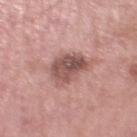Image and clinical context:
From the left lower leg. The recorded lesion diameter is about 5 mm. Captured under white-light illumination. A male patient in their mid- to late 50s. A lesion tile, about 15 mm wide, cut from a 3D total-body photograph.